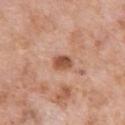Clinical impression: Recorded during total-body skin imaging; not selected for excision or biopsy. Clinical summary: Captured under white-light illumination. The lesion's longest dimension is about 2.5 mm. A female patient aged around 70. The lesion is located on the arm. A 15 mm close-up extracted from a 3D total-body photography capture. Automated tile analysis of the lesion measured border irregularity of about 1.5 on a 0–10 scale, internal color variation of about 3 on a 0–10 scale, and a peripheral color-asymmetry measure near 1.